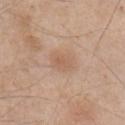The lesion was photographed on a routine skin check and not biopsied; there is no pathology result.
A male subject, roughly 45 years of age.
A 15 mm close-up extracted from a 3D total-body photography capture.
The recorded lesion diameter is about 3 mm.
Captured under white-light illumination.
The total-body-photography lesion software estimated a footprint of about 6.5 mm², a shape eccentricity near 0.65, and a symmetry-axis asymmetry near 0.2. The analysis additionally found an average lesion color of about L≈60 a*≈19 b*≈30 (CIELAB), about 7 CIELAB-L* units darker than the surrounding skin, and a lesion-to-skin contrast of about 5 (normalized; higher = more distinct). The analysis additionally found a border-irregularity rating of about 1.5/10, a within-lesion color-variation index near 2.5/10, and a peripheral color-asymmetry measure near 1. The software also gave a classifier nevus-likeness of about 5/100 and lesion-presence confidence of about 100/100.
From the right upper arm.